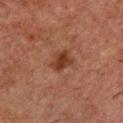• follow-up: no biopsy performed (imaged during a skin exam)
• tile lighting: cross-polarized
• location: the chest
• acquisition: total-body-photography crop, ~15 mm field of view
• subject: male, aged 48–52
• lesion size: ~3 mm (longest diameter)